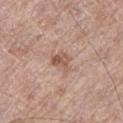Impression:
Part of a total-body skin-imaging series; this lesion was reviewed on a skin check and was not flagged for biopsy.
Context:
From the left thigh. A 15 mm close-up extracted from a 3D total-body photography capture. A male subject, approximately 70 years of age. The total-body-photography lesion software estimated an eccentricity of roughly 0.6 and a shape-asymmetry score of about 0.3 (0 = symmetric). And it measured a border-irregularity rating of about 3/10 and a color-variation rating of about 4.5/10. The software also gave a classifier nevus-likeness of about 5/100 and a lesion-detection confidence of about 100/100.